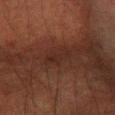Q: Was a biopsy performed?
A: catalogued during a skin exam; not biopsied
Q: What kind of image is this?
A: 15 mm crop, total-body photography
Q: What are the patient's age and sex?
A: male, in their 60s
Q: Lesion size?
A: ≈3.5 mm
Q: What did automated image analysis measure?
A: an outline eccentricity of about 0.9 (0 = round, 1 = elongated) and a symmetry-axis asymmetry near 0.4; a border-irregularity rating of about 5.5/10, a within-lesion color-variation index near 1.5/10, and a peripheral color-asymmetry measure near 0.5
Q: Where on the body is the lesion?
A: the right forearm